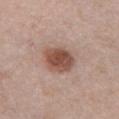Findings:
• size · ~3.5 mm (longest diameter)
• imaging modality · ~15 mm tile from a whole-body skin photo
• lighting · white-light illumination
• image-analysis metrics · border irregularity of about 1.5 on a 0–10 scale and a color-variation rating of about 4/10
• anatomic site · the front of the torso
• subject · female, in their mid-60s